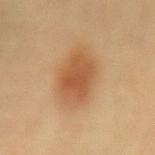follow-up: total-body-photography surveillance lesion; no biopsy | image: total-body-photography crop, ~15 mm field of view | anatomic site: the mid back | patient: female, roughly 45 years of age.